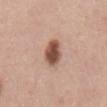Q: Was this lesion biopsied?
A: no biopsy performed (imaged during a skin exam)
Q: What is the imaging modality?
A: ~15 mm tile from a whole-body skin photo
Q: Who is the patient?
A: male, aged approximately 50
Q: How large is the lesion?
A: ≈4 mm
Q: How was the tile lit?
A: white-light illumination
Q: Lesion location?
A: the mid back